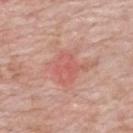| field | value |
|---|---|
| biopsy status | total-body-photography surveillance lesion; no biopsy |
| anatomic site | the mid back |
| patient | male, about 60 years old |
| automated metrics | about 7 CIELAB-L* units darker than the surrounding skin and a lesion-to-skin contrast of about 4.5 (normalized; higher = more distinct) |
| acquisition | 15 mm crop, total-body photography |
| lighting | white-light illumination |
| lesion size | ~7.5 mm (longest diameter) |